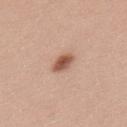Q: Is there a histopathology result?
A: catalogued during a skin exam; not biopsied